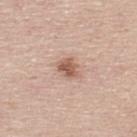Q: What is the imaging modality?
A: 15 mm crop, total-body photography
Q: Lesion location?
A: the upper back
Q: Patient demographics?
A: male, aged 43–47
Q: What did automated image analysis measure?
A: a mean CIELAB color near L≈58 a*≈20 b*≈28, a lesion–skin lightness drop of about 12, and a normalized border contrast of about 8; a border-irregularity rating of about 2.5/10, a within-lesion color-variation index near 3/10, and radial color variation of about 1; a nevus-likeness score of about 85/100 and a detector confidence of about 100 out of 100 that the crop contains a lesion